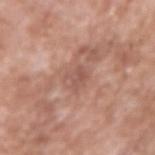Imaged during a routine full-body skin examination; the lesion was not biopsied and no histopathology is available. From the right upper arm. A 15 mm close-up extracted from a 3D total-body photography capture. The patient is a male aged approximately 60. This is a white-light tile. About 3 mm across.